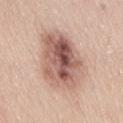{
  "biopsy_status": "not biopsied; imaged during a skin examination",
  "image": {
    "source": "total-body photography crop",
    "field_of_view_mm": 15
  },
  "site": "lower back",
  "patient": {
    "sex": "female",
    "age_approx": 40
  }
}